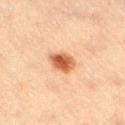Assessment: The lesion was photographed on a routine skin check and not biopsied; there is no pathology result. Context: Located on the right thigh. The patient is a male about 40 years old. The lesion-visualizer software estimated an average lesion color of about L≈49 a*≈24 b*≈33 (CIELAB), roughly 14 lightness units darker than nearby skin, and a normalized lesion–skin contrast near 10. About 3 mm across. Imaged with cross-polarized lighting. A 15 mm close-up tile from a total-body photography series done for melanoma screening.